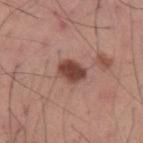biopsy status: imaged on a skin check; not biopsied | TBP lesion metrics: roughly 15 lightness units darker than nearby skin and a lesion-to-skin contrast of about 11 (normalized; higher = more distinct) | subject: male, aged approximately 25 | site: the left upper arm | lesion size: ≈3 mm | tile lighting: white-light | image: 15 mm crop, total-body photography.